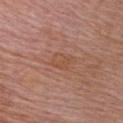{
  "biopsy_status": "not biopsied; imaged during a skin examination",
  "site": "chest",
  "patient": {
    "sex": "male",
    "age_approx": 65
  },
  "image": {
    "source": "total-body photography crop",
    "field_of_view_mm": 15
  }
}